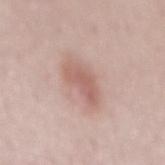Findings:
– follow-up — catalogued during a skin exam; not biopsied
– subject — female, roughly 50 years of age
– illumination — white-light illumination
– automated metrics — a lesion area of about 8 mm² and two-axis asymmetry of about 0.3; a mean CIELAB color near L≈60 a*≈21 b*≈24, roughly 10 lightness units darker than nearby skin, and a normalized lesion–skin contrast near 6.5
– lesion size — ≈4.5 mm
– location — the back
– imaging modality — ~15 mm tile from a whole-body skin photo